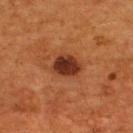Impression:
Captured during whole-body skin photography for melanoma surveillance; the lesion was not biopsied.
Acquisition and patient details:
This is a cross-polarized tile. From the upper back. A region of skin cropped from a whole-body photographic capture, roughly 15 mm wide. The subject is a male roughly 55 years of age.